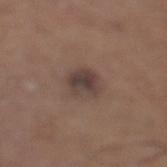biopsy status — catalogued during a skin exam; not biopsied
diameter — ~3 mm (longest diameter)
site — the leg
image source — ~15 mm tile from a whole-body skin photo
patient — male, approximately 65 years of age